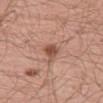Recorded during total-body skin imaging; not selected for excision or biopsy. Automated tile analysis of the lesion measured a footprint of about 5 mm², an eccentricity of roughly 0.7, and two-axis asymmetry of about 0.45. The software also gave a lesion color around L≈52 a*≈23 b*≈29 in CIELAB, roughly 11 lightness units darker than nearby skin, and a normalized border contrast of about 7.5. It also reported a border-irregularity rating of about 4/10, a within-lesion color-variation index near 3.5/10, and peripheral color asymmetry of about 1. From the left thigh. Approximately 3 mm at its widest. Cropped from a whole-body photographic skin survey; the tile spans about 15 mm. A male patient aged 63 to 67.